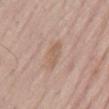Image and clinical context:
The lesion is located on the back. A male patient about 70 years old. Imaged with white-light lighting. The lesion's longest dimension is about 3 mm. A 15 mm close-up extracted from a 3D total-body photography capture.